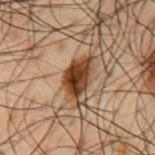Q: Was a biopsy performed?
A: catalogued during a skin exam; not biopsied
Q: What are the patient's age and sex?
A: male, roughly 50 years of age
Q: How large is the lesion?
A: about 4.5 mm
Q: Where on the body is the lesion?
A: the back
Q: What kind of image is this?
A: 15 mm crop, total-body photography